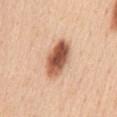<case>
  <biopsy_status>not biopsied; imaged during a skin examination</biopsy_status>
  <patient>
    <sex>female</sex>
    <age_approx>45</age_approx>
  </patient>
  <image>
    <source>total-body photography crop</source>
    <field_of_view_mm>15</field_of_view_mm>
  </image>
  <site>front of the torso</site>
</case>